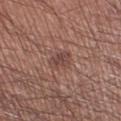• biopsy status — catalogued during a skin exam; not biopsied
• location — the right lower leg
• imaging modality — total-body-photography crop, ~15 mm field of view
• subject — male, aged 43–47